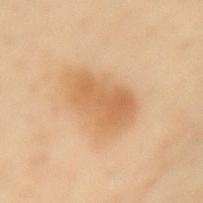This lesion was catalogued during total-body skin photography and was not selected for biopsy. From the mid back. An algorithmic analysis of the crop reported an average lesion color of about L≈55 a*≈18 b*≈35 (CIELAB), roughly 8 lightness units darker than nearby skin, and a lesion-to-skin contrast of about 6.5 (normalized; higher = more distinct). The software also gave border irregularity of about 2.5 on a 0–10 scale, a color-variation rating of about 3/10, and a peripheral color-asymmetry measure near 1. The analysis additionally found a classifier nevus-likeness of about 85/100 and a detector confidence of about 100 out of 100 that the crop contains a lesion. A roughly 15 mm field-of-view crop from a total-body skin photograph. The subject is a female roughly 60 years of age. Imaged with cross-polarized lighting.